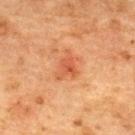Q: Is there a histopathology result?
A: total-body-photography surveillance lesion; no biopsy
Q: Automated lesion metrics?
A: a shape eccentricity near 0.65 and a symmetry-axis asymmetry near 0.4; a lesion color around L≈48 a*≈28 b*≈35 in CIELAB and a lesion-to-skin contrast of about 6 (normalized; higher = more distinct); an automated nevus-likeness rating near 5 out of 100
Q: What is the imaging modality?
A: total-body-photography crop, ~15 mm field of view
Q: Lesion location?
A: the upper back
Q: What are the patient's age and sex?
A: female, aged around 70
Q: What lighting was used for the tile?
A: cross-polarized illumination
Q: How large is the lesion?
A: about 3 mm